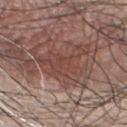Impression:
Captured during whole-body skin photography for melanoma surveillance; the lesion was not biopsied.
Acquisition and patient details:
Located on the chest. A male patient, aged 48 to 52. The tile uses white-light illumination. The recorded lesion diameter is about 6 mm. Automated image analysis of the tile measured a lesion area of about 16 mm², an eccentricity of roughly 0.55, and a shape-asymmetry score of about 0.55 (0 = symmetric). The software also gave border irregularity of about 7.5 on a 0–10 scale, internal color variation of about 3.5 on a 0–10 scale, and peripheral color asymmetry of about 1. A 15 mm close-up tile from a total-body photography series done for melanoma screening.